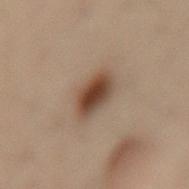The lesion was photographed on a routine skin check and not biopsied; there is no pathology result. A female subject, aged 53 to 57. Measured at roughly 4 mm in maximum diameter. A 15 mm crop from a total-body photograph taken for skin-cancer surveillance. The lesion is located on the lower back. Captured under cross-polarized illumination.